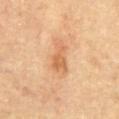Q: Is there a histopathology result?
A: total-body-photography surveillance lesion; no biopsy
Q: How large is the lesion?
A: ~4 mm (longest diameter)
Q: Illumination type?
A: cross-polarized illumination
Q: Lesion location?
A: the front of the torso
Q: What is the imaging modality?
A: total-body-photography crop, ~15 mm field of view
Q: What are the patient's age and sex?
A: male, approximately 85 years of age or older
Q: What did automated image analysis measure?
A: a lesion–skin lightness drop of about 10; a within-lesion color-variation index near 1.5/10 and peripheral color asymmetry of about 0.5